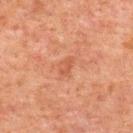The lesion was photographed on a routine skin check and not biopsied; there is no pathology result.
Captured under cross-polarized illumination.
Located on the upper back.
A male subject aged 58 to 62.
An algorithmic analysis of the crop reported a lesion color around L≈42 a*≈22 b*≈29 in CIELAB, roughly 5 lightness units darker than nearby skin, and a lesion-to-skin contrast of about 4.5 (normalized; higher = more distinct).
Longest diameter approximately 2.5 mm.
A lesion tile, about 15 mm wide, cut from a 3D total-body photograph.